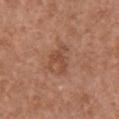biopsy status: no biopsy performed (imaged during a skin exam)
illumination: white-light
lesion size: about 4 mm
body site: the front of the torso
image: 15 mm crop, total-body photography
automated metrics: a color-variation rating of about 1/10 and peripheral color asymmetry of about 0.5; a nevus-likeness score of about 10/100 and lesion-presence confidence of about 100/100
patient: female, roughly 40 years of age